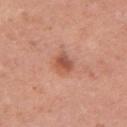<case>
  <biopsy_status>not biopsied; imaged during a skin examination</biopsy_status>
  <lighting>white-light</lighting>
  <automated_metrics>
    <border_irregularity_0_10>2.0</border_irregularity_0_10>
    <peripheral_color_asymmetry>2.0</peripheral_color_asymmetry>
    <nevus_likeness_0_100>80</nevus_likeness_0_100>
    <lesion_detection_confidence_0_100>100</lesion_detection_confidence_0_100>
  </automated_metrics>
  <patient>
    <sex>female</sex>
    <age_approx>35</age_approx>
  </patient>
  <lesion_size>
    <long_diameter_mm_approx>2.5</long_diameter_mm_approx>
  </lesion_size>
  <image>
    <source>total-body photography crop</source>
    <field_of_view_mm>15</field_of_view_mm>
  </image>
  <site>left upper arm</site>
</case>